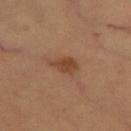| field | value |
|---|---|
| biopsy status | total-body-photography surveillance lesion; no biopsy |
| image | 15 mm crop, total-body photography |
| size | ~3.5 mm (longest diameter) |
| image-analysis metrics | a lesion color around L≈45 a*≈23 b*≈32 in CIELAB and a lesion-to-skin contrast of about 7 (normalized; higher = more distinct); internal color variation of about 3 on a 0–10 scale and radial color variation of about 1.5 |
| tile lighting | cross-polarized illumination |
| patient | female, in their 60s |
| body site | the leg |